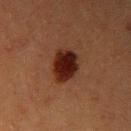Notes:
• workup — catalogued during a skin exam; not biopsied
• diameter — ~4 mm (longest diameter)
• body site — the right upper arm
• tile lighting — cross-polarized
• imaging modality — ~15 mm tile from a whole-body skin photo
• subject — male, aged around 40